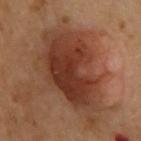Case summary:
* notes · imaged on a skin check; not biopsied
* image-analysis metrics · a border-irregularity index near 5/10, a within-lesion color-variation index near 5.5/10, and peripheral color asymmetry of about 1.5
* lighting · cross-polarized
* subject · female, roughly 60 years of age
* site · the back
* image source · ~15 mm tile from a whole-body skin photo
* size · ~11.5 mm (longest diameter)